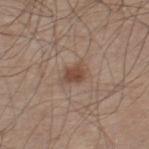biopsy status: no biopsy performed (imaged during a skin exam); tile lighting: white-light; size: ~2.5 mm (longest diameter); patient: male, roughly 60 years of age; body site: the right lower leg; image source: ~15 mm crop, total-body skin-cancer survey.